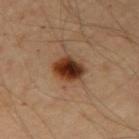biopsy status: total-body-photography surveillance lesion; no biopsy
patient: male, about 65 years old
lesion size: ≈3.5 mm
image: total-body-photography crop, ~15 mm field of view
location: the right upper arm
tile lighting: cross-polarized